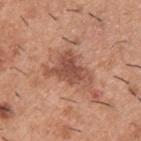Notes:
– notes: no biopsy performed (imaged during a skin exam)
– image source: 15 mm crop, total-body photography
– patient: male, approximately 40 years of age
– TBP lesion metrics: a normalized border contrast of about 8
– lighting: white-light illumination
– body site: the upper back